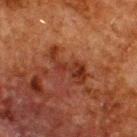lesion diameter: about 5 mm
automated lesion analysis: a color-variation rating of about 3.5/10 and peripheral color asymmetry of about 1; a nevus-likeness score of about 0/100 and a lesion-detection confidence of about 100/100
imaging modality: ~15 mm crop, total-body skin-cancer survey
anatomic site: the upper back
lighting: cross-polarized
patient: male, roughly 60 years of age